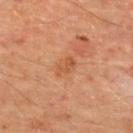Imaged during a routine full-body skin examination; the lesion was not biopsied and no histopathology is available.
From the upper back.
The tile uses cross-polarized illumination.
A male patient, approximately 65 years of age.
Cropped from a total-body skin-imaging series; the visible field is about 15 mm.